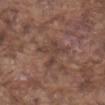{
  "biopsy_status": "not biopsied; imaged during a skin examination",
  "site": "front of the torso",
  "lighting": "white-light",
  "patient": {
    "sex": "male",
    "age_approx": 75
  },
  "automated_metrics": {
    "area_mm2_approx": 8.5,
    "eccentricity": 0.6,
    "cielab_L": 42,
    "cielab_a": 18,
    "cielab_b": 23,
    "vs_skin_darker_L": 7.0,
    "vs_skin_contrast_norm": 5.5,
    "nevus_likeness_0_100": 0
  },
  "image": {
    "source": "total-body photography crop",
    "field_of_view_mm": 15
  }
}